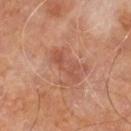Q: Is there a histopathology result?
A: imaged on a skin check; not biopsied
Q: Who is the patient?
A: male, aged approximately 60
Q: What is the imaging modality?
A: 15 mm crop, total-body photography
Q: Where on the body is the lesion?
A: the right leg
Q: Automated lesion metrics?
A: roughly 7 lightness units darker than nearby skin; border irregularity of about 6.5 on a 0–10 scale, a within-lesion color-variation index near 0.5/10, and a peripheral color-asymmetry measure near 0; lesion-presence confidence of about 100/100
Q: Illumination type?
A: cross-polarized illumination
Q: What is the lesion's diameter?
A: ~4 mm (longest diameter)